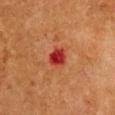No biopsy was performed on this lesion — it was imaged during a full skin examination and was not determined to be concerning.
This is a cross-polarized tile.
The lesion is on the chest.
Approximately 3 mm at its widest.
The subject is a female aged around 50.
The lesion-visualizer software estimated a footprint of about 4.5 mm² and two-axis asymmetry of about 0.25.
A region of skin cropped from a whole-body photographic capture, roughly 15 mm wide.